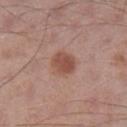{
  "biopsy_status": "not biopsied; imaged during a skin examination",
  "automated_metrics": {
    "eccentricity": 0.45,
    "shape_asymmetry": 0.15,
    "cielab_L": 50,
    "cielab_a": 22,
    "cielab_b": 27,
    "vs_skin_darker_L": 10.0,
    "vs_skin_contrast_norm": 8.0,
    "nevus_likeness_0_100": 95,
    "lesion_detection_confidence_0_100": 100
  },
  "site": "left lower leg",
  "patient": {
    "sex": "male",
    "age_approx": 55
  },
  "lesion_size": {
    "long_diameter_mm_approx": 3.0
  },
  "image": {
    "source": "total-body photography crop",
    "field_of_view_mm": 15
  }
}